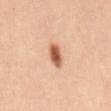Imaged during a routine full-body skin examination; the lesion was not biopsied and no histopathology is available.
A female patient, in their 40s.
A lesion tile, about 15 mm wide, cut from a 3D total-body photograph.
From the mid back.
The lesion's longest dimension is about 3.5 mm.